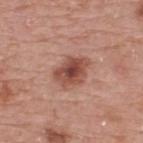Imaged during a routine full-body skin examination; the lesion was not biopsied and no histopathology is available. From the upper back. Cropped from a total-body skin-imaging series; the visible field is about 15 mm. Longest diameter approximately 4 mm. The tile uses white-light illumination. A male patient aged around 75.